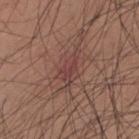Impression: Imaged during a routine full-body skin examination; the lesion was not biopsied and no histopathology is available. Acquisition and patient details: The total-body-photography lesion software estimated a lesion area of about 6 mm², a shape eccentricity near 0.9, and two-axis asymmetry of about 0.5. The analysis additionally found border irregularity of about 5 on a 0–10 scale and a color-variation rating of about 2.5/10. The analysis additionally found a classifier nevus-likeness of about 5/100. About 4 mm across. Imaged with white-light lighting. The patient is a male aged approximately 35. Located on the mid back. A close-up tile cropped from a whole-body skin photograph, about 15 mm across.